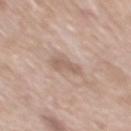<lesion>
  <biopsy_status>not biopsied; imaged during a skin examination</biopsy_status>
  <image>
    <source>total-body photography crop</source>
    <field_of_view_mm>15</field_of_view_mm>
  </image>
  <patient>
    <sex>male</sex>
    <age_approx>60</age_approx>
  </patient>
  <site>mid back</site>
</lesion>